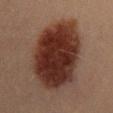Case summary:
* follow-up · total-body-photography surveillance lesion; no biopsy
* image-analysis metrics · an area of roughly 49 mm², an eccentricity of roughly 0.8, and a shape-asymmetry score of about 0.15 (0 = symmetric); a lesion-detection confidence of about 100/100
* location · the mid back
* size · ≈10 mm
* subject · male, aged approximately 60
* image · ~15 mm crop, total-body skin-cancer survey